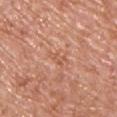biopsy_status: not biopsied; imaged during a skin examination
lesion_size:
  long_diameter_mm_approx: 2.5
image:
  source: total-body photography crop
  field_of_view_mm: 15
automated_metrics:
  border_irregularity_0_10: 5.0
  color_variation_0_10: 0.0
  peripheral_color_asymmetry: 0.0
site: chest
patient:
  sex: male
  age_approx: 70